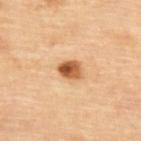{
  "biopsy_status": "not biopsied; imaged during a skin examination",
  "patient": {
    "sex": "female",
    "age_approx": 65
  },
  "site": "upper back",
  "automated_metrics": {
    "area_mm2_approx": 5.5,
    "eccentricity": 0.7,
    "nevus_likeness_0_100": 100,
    "lesion_detection_confidence_0_100": 100
  },
  "lighting": "cross-polarized",
  "image": {
    "source": "total-body photography crop",
    "field_of_view_mm": 15
  }
}